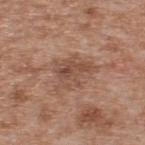follow-up=catalogued during a skin exam; not biopsied.